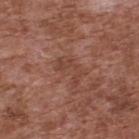biopsy_status: not biopsied; imaged during a skin examination
lighting: white-light
site: upper back
lesion_size:
  long_diameter_mm_approx: 3.5
image:
  source: total-body photography crop
  field_of_view_mm: 15
patient:
  sex: male
  age_approx: 75